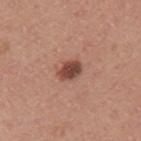Notes:
- biopsy status · no biopsy performed (imaged during a skin exam)
- image source · ~15 mm crop, total-body skin-cancer survey
- subject · male, approximately 40 years of age
- diameter · ≈3 mm
- lighting · white-light illumination
- location · the arm
- TBP lesion metrics · a lesion area of about 5 mm² and an outline eccentricity of about 0.75 (0 = round, 1 = elongated); a lesion color around L≈45 a*≈23 b*≈26 in CIELAB, roughly 15 lightness units darker than nearby skin, and a normalized border contrast of about 10.5; a border-irregularity index near 2/10 and a peripheral color-asymmetry measure near 1; a classifier nevus-likeness of about 80/100